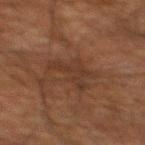Captured during whole-body skin photography for melanoma surveillance; the lesion was not biopsied. The tile uses cross-polarized illumination. Located on the mid back. A male subject, aged approximately 60. Longest diameter approximately 4 mm. Cropped from a whole-body photographic skin survey; the tile spans about 15 mm.